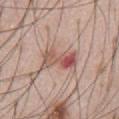Clinical summary:
This is a white-light tile. Automated image analysis of the tile measured a mean CIELAB color near L≈56 a*≈22 b*≈25 and roughly 11 lightness units darker than nearby skin. And it measured a color-variation rating of about 10/10 and peripheral color asymmetry of about 6. It also reported an automated nevus-likeness rating near 0 out of 100. A region of skin cropped from a whole-body photographic capture, roughly 15 mm wide. A male subject, aged 58–62. The lesion's longest dimension is about 5 mm. From the abdomen.